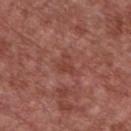{"biopsy_status": "not biopsied; imaged during a skin examination", "image": {"source": "total-body photography crop", "field_of_view_mm": 15}, "automated_metrics": {"area_mm2_approx": 3.0, "eccentricity": 0.6, "border_irregularity_0_10": 6.5, "color_variation_0_10": 0.5, "peripheral_color_asymmetry": 0.5, "nevus_likeness_0_100": 0}, "lighting": "white-light", "site": "front of the torso", "patient": {"sex": "male", "age_approx": 65}}